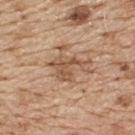From the upper back. Measured at roughly 5 mm in maximum diameter. A 15 mm crop from a total-body photograph taken for skin-cancer surveillance. Automated tile analysis of the lesion measured an area of roughly 9 mm². The analysis additionally found an average lesion color of about L≈55 a*≈19 b*≈32 (CIELAB), roughly 10 lightness units darker than nearby skin, and a normalized lesion–skin contrast near 7. And it measured a nevus-likeness score of about 0/100 and a detector confidence of about 95 out of 100 that the crop contains a lesion. This is a white-light tile. The subject is a male in their 70s.